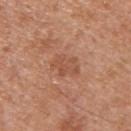<record>
<biopsy_status>not biopsied; imaged during a skin examination</biopsy_status>
<patient>
  <sex>male</sex>
  <age_approx>30</age_approx>
</patient>
<lesion_size>
  <long_diameter_mm_approx>3.5</long_diameter_mm_approx>
</lesion_size>
<site>upper back</site>
<automated_metrics>
  <area_mm2_approx>6.5</area_mm2_approx>
  <eccentricity>0.75</eccentricity>
  <shape_asymmetry>0.3</shape_asymmetry>
  <border_irregularity_0_10>3.5</border_irregularity_0_10>
  <peripheral_color_asymmetry>0.5</peripheral_color_asymmetry>
</automated_metrics>
<lighting>white-light</lighting>
<image>
  <source>total-body photography crop</source>
  <field_of_view_mm>15</field_of_view_mm>
</image>
</record>